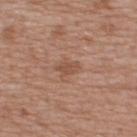Case summary:
* workup — catalogued during a skin exam; not biopsied
* anatomic site — the upper back
* patient — male, aged around 65
* lesion size — about 2.5 mm
* lighting — white-light
* image — total-body-photography crop, ~15 mm field of view